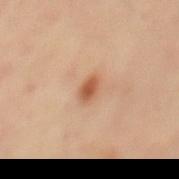Context:
The recorded lesion diameter is about 2.5 mm. Imaged with cross-polarized lighting. A male patient about 50 years old. From the mid back. A close-up tile cropped from a whole-body skin photograph, about 15 mm across.